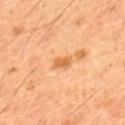Case summary:
* workup — total-body-photography surveillance lesion; no biopsy
* lesion diameter — ≈2.5 mm
* subject — male, aged 58–62
* imaging modality — total-body-photography crop, ~15 mm field of view
* lighting — cross-polarized
* anatomic site — the upper back